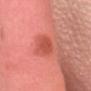biopsy_status: not biopsied; imaged during a skin examination
patient:
  sex: female
  age_approx: 45
image:
  source: total-body photography crop
  field_of_view_mm: 15
site: head or neck
lesion_size:
  long_diameter_mm_approx: 3.5